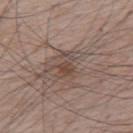Clinical impression:
Captured during whole-body skin photography for melanoma surveillance; the lesion was not biopsied.
Background:
A male patient, in their mid-60s. A close-up tile cropped from a whole-body skin photograph, about 15 mm across. This is a white-light tile. The lesion-visualizer software estimated a normalized border contrast of about 6.5. It also reported a color-variation rating of about 2.5/10 and radial color variation of about 1. Located on the upper back.